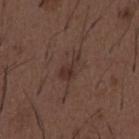| field | value |
|---|---|
| workup | catalogued during a skin exam; not biopsied |
| lighting | white-light |
| patient | male, aged approximately 50 |
| acquisition | 15 mm crop, total-body photography |
| automated metrics | roughly 7 lightness units darker than nearby skin and a normalized border contrast of about 7; a border-irregularity rating of about 5.5/10, a within-lesion color-variation index near 2/10, and radial color variation of about 0.5 |
| diameter | ≈3.5 mm |
| anatomic site | the abdomen |